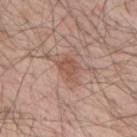Recorded during total-body skin imaging; not selected for excision or biopsy.